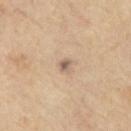- workup · imaged on a skin check; not biopsied
- illumination · cross-polarized
- diameter · ~1.5 mm (longest diameter)
- anatomic site · the front of the torso
- acquisition · total-body-photography crop, ~15 mm field of view